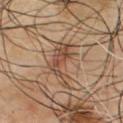Captured during whole-body skin photography for melanoma surveillance; the lesion was not biopsied.
A close-up tile cropped from a whole-body skin photograph, about 15 mm across.
About 5.5 mm across.
Imaged with cross-polarized lighting.
A male patient, roughly 65 years of age.
The lesion is on the chest.